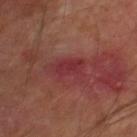<record>
<image>
  <source>total-body photography crop</source>
  <field_of_view_mm>15</field_of_view_mm>
</image>
<automated_metrics>
  <area_mm2_approx>8.5</area_mm2_approx>
  <eccentricity>0.8</eccentricity>
  <shape_asymmetry>0.4</shape_asymmetry>
  <border_irregularity_0_10>5.0</border_irregularity_0_10>
  <color_variation_0_10>3.0</color_variation_0_10>
  <peripheral_color_asymmetry>1.0</peripheral_color_asymmetry>
  <nevus_likeness_0_100>0</nevus_likeness_0_100>
  <lesion_detection_confidence_0_100>80</lesion_detection_confidence_0_100>
</automated_metrics>
<lesion_size>
  <long_diameter_mm_approx>4.0</long_diameter_mm_approx>
</lesion_size>
<site>right lower leg</site>
<patient>
  <sex>male</sex>
  <age_approx>70</age_approx>
</patient>
</record>